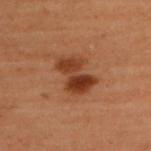Case summary:
• biopsy status · imaged on a skin check; not biopsied
• lesion diameter · about 4.5 mm
• image · total-body-photography crop, ~15 mm field of view
• illumination · cross-polarized illumination
• anatomic site · the upper back
• patient · female, aged approximately 50